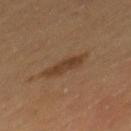The lesion was photographed on a routine skin check and not biopsied; there is no pathology result. Cropped from a total-body skin-imaging series; the visible field is about 15 mm. A male patient, about 60 years old. On the mid back. Automated image analysis of the tile measured an area of roughly 6.5 mm², a shape eccentricity near 0.9, and a shape-asymmetry score of about 0.2 (0 = symmetric). It also reported a lesion color around L≈34 a*≈16 b*≈27 in CIELAB and roughly 7 lightness units darker than nearby skin. And it measured a classifier nevus-likeness of about 65/100 and lesion-presence confidence of about 100/100.